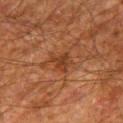| field | value |
|---|---|
| workup | no biopsy performed (imaged during a skin exam) |
| patient | male, aged 78 to 82 |
| site | the leg |
| automated lesion analysis | a lesion color around L≈31 a*≈20 b*≈28 in CIELAB and a lesion–skin lightness drop of about 7 |
| tile lighting | cross-polarized |
| image | total-body-photography crop, ~15 mm field of view |